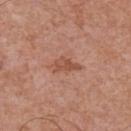Findings:
• follow-up — no biopsy performed (imaged during a skin exam)
• patient — male, aged 68 to 72
• image — 15 mm crop, total-body photography
• site — the chest
• illumination — white-light